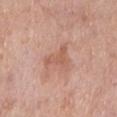  biopsy_status: not biopsied; imaged during a skin examination
  patient:
    sex: female
    age_approx: 55
  image:
    source: total-body photography crop
    field_of_view_mm: 15
  site: leg
  lesion_size:
    long_diameter_mm_approx: 4.0